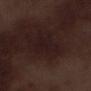A male patient, roughly 70 years of age. On the left lower leg. A lesion tile, about 15 mm wide, cut from a 3D total-body photograph.